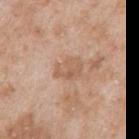Q: Was this lesion biopsied?
A: no biopsy performed (imaged during a skin exam)
Q: Lesion location?
A: the arm
Q: Patient demographics?
A: male, aged 48–52
Q: What kind of image is this?
A: total-body-photography crop, ~15 mm field of view